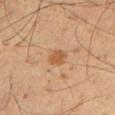This lesion was catalogued during total-body skin photography and was not selected for biopsy.
A male subject, aged around 50.
The recorded lesion diameter is about 3 mm.
Automated tile analysis of the lesion measured a border-irregularity rating of about 2/10, a within-lesion color-variation index near 2.5/10, and a peripheral color-asymmetry measure near 1. The analysis additionally found a detector confidence of about 100 out of 100 that the crop contains a lesion.
This is a cross-polarized tile.
A 15 mm close-up tile from a total-body photography series done for melanoma screening.
From the right upper arm.